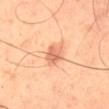notes = catalogued during a skin exam; not biopsied.